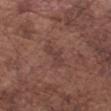Assessment: Imaged during a routine full-body skin examination; the lesion was not biopsied and no histopathology is available. Background: On the right forearm. The subject is a male approximately 75 years of age. A lesion tile, about 15 mm wide, cut from a 3D total-body photograph.